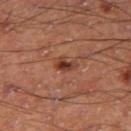Clinical impression: The lesion was photographed on a routine skin check and not biopsied; there is no pathology result. Context: On the right thigh. Longest diameter approximately 2.5 mm. This is a cross-polarized tile. Automated image analysis of the tile measured an outline eccentricity of about 0.8 (0 = round, 1 = elongated) and a symmetry-axis asymmetry near 0.4. The software also gave a border-irregularity index near 4/10, a color-variation rating of about 2.5/10, and a peripheral color-asymmetry measure near 0.5. A male subject, approximately 60 years of age. Cropped from a total-body skin-imaging series; the visible field is about 15 mm.